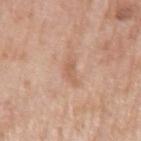notes: imaged on a skin check; not biopsied
acquisition: ~15 mm crop, total-body skin-cancer survey
subject: male, about 70 years old
location: the left upper arm